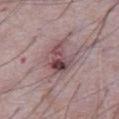* follow-up: imaged on a skin check; not biopsied
* patient: male, approximately 65 years of age
* lesion size: about 4 mm
* image source: total-body-photography crop, ~15 mm field of view
* location: the abdomen